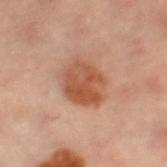Acquisition and patient details: A female subject aged 58–62. This is a cross-polarized tile. A 15 mm close-up extracted from a 3D total-body photography capture. Located on the left leg. An algorithmic analysis of the crop reported a footprint of about 15 mm², an outline eccentricity of about 0.5 (0 = round, 1 = elongated), and a shape-asymmetry score of about 0.2 (0 = symmetric). The analysis additionally found an average lesion color of about L≈46 a*≈22 b*≈30 (CIELAB), a lesion–skin lightness drop of about 10, and a normalized border contrast of about 8.5. The analysis additionally found a border-irregularity index near 2/10, a color-variation rating of about 5.5/10, and radial color variation of about 2. The analysis additionally found a classifier nevus-likeness of about 100/100 and a lesion-detection confidence of about 100/100.